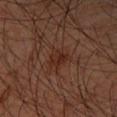A close-up tile cropped from a whole-body skin photograph, about 15 mm across. On the left forearm. The subject is a male in their 60s. Imaged with cross-polarized lighting.